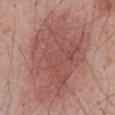Notes:
- biopsy status — imaged on a skin check; not biopsied
- patient — male, aged 68 to 72
- lighting — white-light illumination
- acquisition — ~15 mm tile from a whole-body skin photo
- size — ~12 mm (longest diameter)
- body site — the abdomen